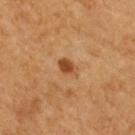Background:
The tile uses cross-polarized illumination. A lesion tile, about 15 mm wide, cut from a 3D total-body photograph. A female subject aged 63 to 67. The lesion is on the upper back. Measured at roughly 2.5 mm in maximum diameter.